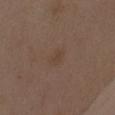Clinical impression:
Part of a total-body skin-imaging series; this lesion was reviewed on a skin check and was not flagged for biopsy.
Image and clinical context:
Located on the chest. The subject is a female aged 33 to 37. Measured at roughly 2.5 mm in maximum diameter. Automated image analysis of the tile measured a lesion area of about 3 mm² and a symmetry-axis asymmetry near 0.35. It also reported internal color variation of about 1.5 on a 0–10 scale and a peripheral color-asymmetry measure near 0.5. A 15 mm close-up extracted from a 3D total-body photography capture. This is a white-light tile.